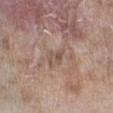The lesion was tiled from a total-body skin photograph and was not biopsied.
From the right lower leg.
Cropped from a whole-body photographic skin survey; the tile spans about 15 mm.
The lesion-visualizer software estimated an eccentricity of roughly 0.8 and a symmetry-axis asymmetry near 0.5. The analysis additionally found a detector confidence of about 85 out of 100 that the crop contains a lesion.
The patient is a male aged 58 to 62.